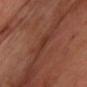Impression:
The lesion was tiled from a total-body skin photograph and was not biopsied.
Image and clinical context:
The lesion is located on the chest. A male subject, aged 68 to 72. The tile uses cross-polarized illumination. Measured at roughly 2.5 mm in maximum diameter. The total-body-photography lesion software estimated a lesion–skin lightness drop of about 5 and a normalized lesion–skin contrast near 4.5. The software also gave a within-lesion color-variation index near 1.5/10 and a peripheral color-asymmetry measure near 0.5. The software also gave a nevus-likeness score of about 0/100 and lesion-presence confidence of about 70/100. A roughly 15 mm field-of-view crop from a total-body skin photograph.